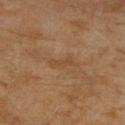<lesion>
<biopsy_status>not biopsied; imaged during a skin examination</biopsy_status>
<image>
  <source>total-body photography crop</source>
  <field_of_view_mm>15</field_of_view_mm>
</image>
<site>right upper arm</site>
<lighting>cross-polarized</lighting>
<lesion_size>
  <long_diameter_mm_approx>3.0</long_diameter_mm_approx>
</lesion_size>
<patient>
  <sex>female</sex>
  <age_approx>60</age_approx>
</patient>
</lesion>